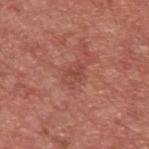biopsy_status: not biopsied; imaged during a skin examination
lesion_size:
  long_diameter_mm_approx: 2.5
image:
  source: total-body photography crop
  field_of_view_mm: 15
lighting: white-light
site: upper back
patient:
  sex: male
  age_approx: 65